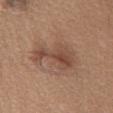workup: catalogued during a skin exam; not biopsied
site: the chest
image source: ~15 mm crop, total-body skin-cancer survey
diameter: ≈5.5 mm
subject: male, roughly 60 years of age